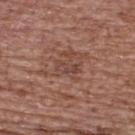The lesion was tiled from a total-body skin photograph and was not biopsied. Measured at roughly 3 mm in maximum diameter. A female patient, aged approximately 65. A lesion tile, about 15 mm wide, cut from a 3D total-body photograph. Imaged with white-light lighting. Located on the upper back.